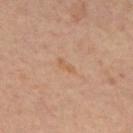Imaged during a routine full-body skin examination; the lesion was not biopsied and no histopathology is available. A lesion tile, about 15 mm wide, cut from a 3D total-body photograph. Longest diameter approximately 2.5 mm. A female subject, aged 43–47. This is a cross-polarized tile. Located on the upper back.